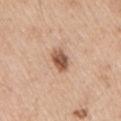No biopsy was performed on this lesion — it was imaged during a full skin examination and was not determined to be concerning.
A male patient, about 65 years old.
Located on the right upper arm.
A lesion tile, about 15 mm wide, cut from a 3D total-body photograph.